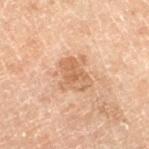Image and clinical context:
The patient is a male roughly 70 years of age. A 15 mm close-up tile from a total-body photography series done for melanoma screening. On the right lower leg. The recorded lesion diameter is about 4 mm. The lesion-visualizer software estimated a border-irregularity index near 3.5/10 and internal color variation of about 3.5 on a 0–10 scale.